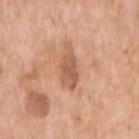Q: Was this lesion biopsied?
A: total-body-photography surveillance lesion; no biopsy
Q: Lesion location?
A: the arm
Q: Who is the patient?
A: female, aged 73 to 77
Q: What is the imaging modality?
A: total-body-photography crop, ~15 mm field of view